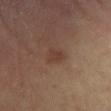The lesion was photographed on a routine skin check and not biopsied; there is no pathology result. The patient is a male aged approximately 65. This is a cross-polarized tile. Cropped from a whole-body photographic skin survey; the tile spans about 15 mm.